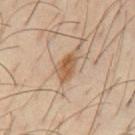| key | value |
|---|---|
| follow-up | imaged on a skin check; not biopsied |
| lesion diameter | ≈4 mm |
| patient | male, about 40 years old |
| illumination | cross-polarized |
| acquisition | total-body-photography crop, ~15 mm field of view |
| site | the chest |